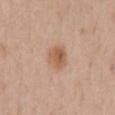Image and clinical context: A 15 mm close-up tile from a total-body photography series done for melanoma screening. Captured under white-light illumination. On the chest. The subject is a male about 55 years old. About 3 mm across.